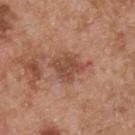Q: Was this lesion biopsied?
A: no biopsy performed (imaged during a skin exam)
Q: What kind of image is this?
A: ~15 mm crop, total-body skin-cancer survey
Q: How was the tile lit?
A: white-light
Q: Lesion size?
A: ≈4 mm
Q: What is the anatomic site?
A: the upper back
Q: What are the patient's age and sex?
A: male, aged 53–57